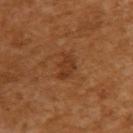image-analysis metrics — a border-irregularity rating of about 3/10, internal color variation of about 2 on a 0–10 scale, and a peripheral color-asymmetry measure near 0.5; a classifier nevus-likeness of about 5/100
image source — ~15 mm tile from a whole-body skin photo
lesion size — ≈3 mm
subject — female, about 55 years old
location — the back